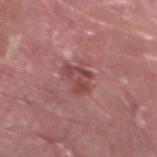{
  "biopsy_status": "not biopsied; imaged during a skin examination",
  "site": "left lower leg",
  "lesion_size": {
    "long_diameter_mm_approx": 3.5
  },
  "lighting": "white-light",
  "patient": {
    "sex": "male",
    "age_approx": 40
  },
  "image": {
    "source": "total-body photography crop",
    "field_of_view_mm": 15
  }
}